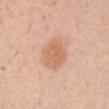<tbp_lesion>
  <biopsy_status>not biopsied; imaged during a skin examination</biopsy_status>
  <image>
    <source>total-body photography crop</source>
    <field_of_view_mm>15</field_of_view_mm>
  </image>
  <patient>
    <sex>female</sex>
    <age_approx>20</age_approx>
  </patient>
  <lighting>white-light</lighting>
  <site>right upper arm</site>
</tbp_lesion>